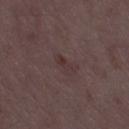Clinical impression: Part of a total-body skin-imaging series; this lesion was reviewed on a skin check and was not flagged for biopsy. Context: A close-up tile cropped from a whole-body skin photograph, about 15 mm across. A male patient, aged around 50.